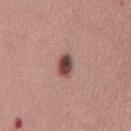  biopsy_status: not biopsied; imaged during a skin examination
  lighting: white-light
  site: chest
  image:
    source: total-body photography crop
    field_of_view_mm: 15
  patient:
    sex: female
    age_approx: 35
  lesion_size:
    long_diameter_mm_approx: 2.5